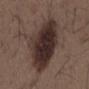Impression:
The lesion was tiled from a total-body skin photograph and was not biopsied.
Image and clinical context:
Cropped from a total-body skin-imaging series; the visible field is about 15 mm. The subject is a male about 50 years old. On the chest.